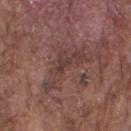Context: Automated tile analysis of the lesion measured a footprint of about 10 mm² and a shape eccentricity near 0.9. It also reported a border-irregularity index near 9/10 and a within-lesion color-variation index near 2.5/10. A roughly 15 mm field-of-view crop from a total-body skin photograph. The tile uses white-light illumination. The lesion is located on the right upper arm. Measured at roughly 6.5 mm in maximum diameter. A male patient in their mid-70s.